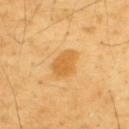Q: Was this lesion biopsied?
A: catalogued during a skin exam; not biopsied
Q: Illumination type?
A: cross-polarized illumination
Q: Lesion location?
A: the upper back
Q: What are the patient's age and sex?
A: male, aged approximately 65
Q: How large is the lesion?
A: ≈4 mm
Q: What kind of image is this?
A: 15 mm crop, total-body photography
Q: Automated lesion metrics?
A: border irregularity of about 2 on a 0–10 scale, internal color variation of about 2 on a 0–10 scale, and a peripheral color-asymmetry measure near 0.5; an automated nevus-likeness rating near 45 out of 100 and a lesion-detection confidence of about 100/100